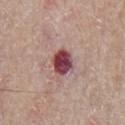Part of a total-body skin-imaging series; this lesion was reviewed on a skin check and was not flagged for biopsy.
A 15 mm close-up extracted from a 3D total-body photography capture.
A male subject about 65 years old.
The lesion is located on the chest.
The tile uses white-light illumination.